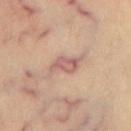This lesion was catalogued during total-body skin photography and was not selected for biopsy.
A region of skin cropped from a whole-body photographic capture, roughly 15 mm wide.
The lesion is on the chest.
The recorded lesion diameter is about 4.5 mm.